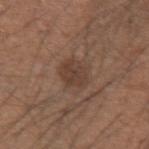{
  "biopsy_status": "not biopsied; imaged during a skin examination"
}